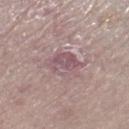notes=catalogued during a skin exam; not biopsied | anatomic site=the right lower leg | imaging modality=~15 mm crop, total-body skin-cancer survey | patient=female, roughly 70 years of age.